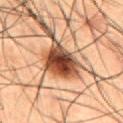Assessment:
The lesion was photographed on a routine skin check and not biopsied; there is no pathology result.
Acquisition and patient details:
About 4.5 mm across. A lesion tile, about 15 mm wide, cut from a 3D total-body photograph. The lesion is on the abdomen. The patient is a male approximately 50 years of age. This is a cross-polarized tile.